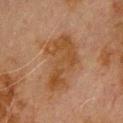follow-up — catalogued during a skin exam; not biopsied | size — ~6.5 mm (longest diameter) | image-analysis metrics — a lesion color around L≈37 a*≈17 b*≈30 in CIELAB and a lesion–skin lightness drop of about 6; a classifier nevus-likeness of about 0/100 and a detector confidence of about 100 out of 100 that the crop contains a lesion | tile lighting — cross-polarized illumination | anatomic site — the upper back | subject — male, aged 78 to 82 | acquisition — ~15 mm tile from a whole-body skin photo.